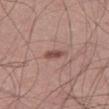– follow-up: catalogued during a skin exam; not biopsied
– imaging modality: ~15 mm tile from a whole-body skin photo
– image-analysis metrics: a footprint of about 3 mm², a shape eccentricity near 0.85, and two-axis asymmetry of about 0.2; a border-irregularity index near 2/10, a color-variation rating of about 1.5/10, and peripheral color asymmetry of about 0.5
– patient: male, approximately 55 years of age
– site: the right thigh
– lighting: white-light